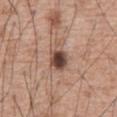| key | value |
|---|---|
| follow-up | catalogued during a skin exam; not biopsied |
| image source | ~15 mm tile from a whole-body skin photo |
| automated metrics | a lesion area of about 6.5 mm², an eccentricity of roughly 0.75, and a symmetry-axis asymmetry near 0.25; a border-irregularity index near 2.5/10 and peripheral color asymmetry of about 1.5; an automated nevus-likeness rating near 80 out of 100 |
| anatomic site | the abdomen |
| subject | male, aged approximately 60 |